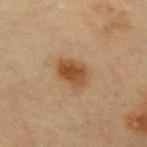Imaged during a routine full-body skin examination; the lesion was not biopsied and no histopathology is available. A 15 mm crop from a total-body photograph taken for skin-cancer surveillance. The subject is a male in their mid- to late 60s. Located on the right upper arm.